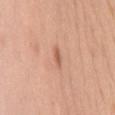Findings:
– workup — total-body-photography surveillance lesion; no biopsy
– size — ~2.5 mm (longest diameter)
– anatomic site — the mid back
– lighting — white-light
– patient — female, in their 50s
– acquisition — ~15 mm tile from a whole-body skin photo